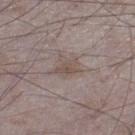This lesion was catalogued during total-body skin photography and was not selected for biopsy. A close-up tile cropped from a whole-body skin photograph, about 15 mm across. From the leg. Captured under white-light illumination. A male patient roughly 75 years of age. Measured at roughly 3 mm in maximum diameter.